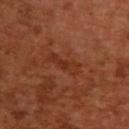On the upper back. A female subject aged around 55. Imaged with cross-polarized lighting. A roughly 15 mm field-of-view crop from a total-body skin photograph. The lesion's longest dimension is about 4 mm.